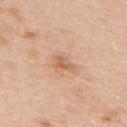follow-up: imaged on a skin check; not biopsied
diameter: about 2.5 mm
patient: female, about 45 years old
image source: ~15 mm crop, total-body skin-cancer survey
tile lighting: white-light
body site: the upper back